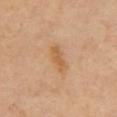| field | value |
|---|---|
| follow-up | imaged on a skin check; not biopsied |
| patient | male, aged 63–67 |
| lighting | cross-polarized |
| automated lesion analysis | a lesion area of about 4.5 mm², a shape eccentricity near 0.9, and a symmetry-axis asymmetry near 0.25; a classifier nevus-likeness of about 40/100 |
| image | total-body-photography crop, ~15 mm field of view |
| site | the chest |